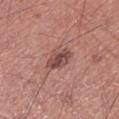This lesion was catalogued during total-body skin photography and was not selected for biopsy. A close-up tile cropped from a whole-body skin photograph, about 15 mm across. Captured under white-light illumination. The subject is a male aged around 60. The lesion-visualizer software estimated a lesion color around L≈48 a*≈23 b*≈23 in CIELAB, about 11 CIELAB-L* units darker than the surrounding skin, and a lesion-to-skin contrast of about 8 (normalized; higher = more distinct). The software also gave a classifier nevus-likeness of about 35/100 and a lesion-detection confidence of about 100/100. About 3.5 mm across. From the left lower leg.